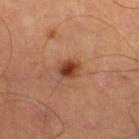notes: total-body-photography surveillance lesion; no biopsy | acquisition: 15 mm crop, total-body photography | automated metrics: an average lesion color of about L≈38 a*≈23 b*≈31 (CIELAB), about 12 CIELAB-L* units darker than the surrounding skin, and a normalized border contrast of about 10; a border-irregularity rating of about 1.5/10, internal color variation of about 4.5 on a 0–10 scale, and a peripheral color-asymmetry measure near 1.5; an automated nevus-likeness rating near 100 out of 100 and a detector confidence of about 100 out of 100 that the crop contains a lesion | body site: the left thigh | subject: male, roughly 65 years of age | diameter: ~2.5 mm (longest diameter).